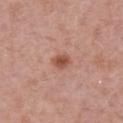From the back. Imaged with white-light lighting. Automated tile analysis of the lesion measured a footprint of about 3.5 mm² and an outline eccentricity of about 0.55 (0 = round, 1 = elongated). The analysis additionally found an average lesion color of about L≈52 a*≈25 b*≈30 (CIELAB) and a normalized border contrast of about 8.5. The analysis additionally found an automated nevus-likeness rating near 95 out of 100. The recorded lesion diameter is about 2 mm. A female subject approximately 65 years of age. A lesion tile, about 15 mm wide, cut from a 3D total-body photograph.